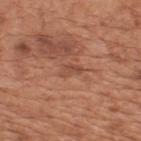The lesion was tiled from a total-body skin photograph and was not biopsied. The lesion is on the upper back. Imaged with white-light lighting. A male subject in their mid-60s. Measured at roughly 3 mm in maximum diameter. The lesion-visualizer software estimated a detector confidence of about 85 out of 100 that the crop contains a lesion. A roughly 15 mm field-of-view crop from a total-body skin photograph.